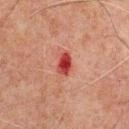This lesion was catalogued during total-body skin photography and was not selected for biopsy. A region of skin cropped from a whole-body photographic capture, roughly 15 mm wide. A male subject about 60 years old. The lesion is located on the chest. Approximately 3 mm at its widest.